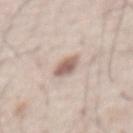Notes:
• notes · no biopsy performed (imaged during a skin exam)
• subject · male, about 50 years old
• lesion diameter · about 3 mm
• lighting · white-light illumination
• imaging modality · 15 mm crop, total-body photography
• site · the chest